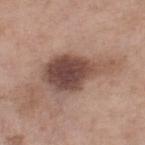Q: How large is the lesion?
A: ~8 mm (longest diameter)
Q: What kind of image is this?
A: ~15 mm tile from a whole-body skin photo
Q: What are the patient's age and sex?
A: female, aged approximately 55
Q: What is the anatomic site?
A: the right lower leg
Q: Illumination type?
A: white-light illumination